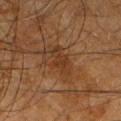  biopsy_status: not biopsied; imaged during a skin examination
  patient:
    sex: male
    age_approx: 65
  image:
    source: total-body photography crop
    field_of_view_mm: 15
  site: left lower leg